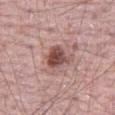Clinical impression:
Imaged during a routine full-body skin examination; the lesion was not biopsied and no histopathology is available.
Clinical summary:
On the leg. This is a white-light tile. A male patient, aged around 70. A lesion tile, about 15 mm wide, cut from a 3D total-body photograph.